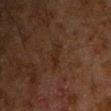Captured during whole-body skin photography for melanoma surveillance; the lesion was not biopsied. Captured under cross-polarized illumination. A male subject aged 58–62. A close-up tile cropped from a whole-body skin photograph, about 15 mm across. The total-body-photography lesion software estimated a lesion color around L≈18 a*≈14 b*≈20 in CIELAB, about 4 CIELAB-L* units darker than the surrounding skin, and a lesion-to-skin contrast of about 5.5 (normalized; higher = more distinct). It also reported a border-irregularity index near 4/10, internal color variation of about 0 on a 0–10 scale, and a peripheral color-asymmetry measure near 0. The recorded lesion diameter is about 3 mm. Located on the chest.